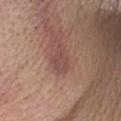This lesion was catalogued during total-body skin photography and was not selected for biopsy.
A male subject in their mid-50s.
Cropped from a whole-body photographic skin survey; the tile spans about 15 mm.
From the head or neck.